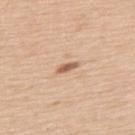<lesion>
  <image>
    <source>total-body photography crop</source>
    <field_of_view_mm>15</field_of_view_mm>
  </image>
  <site>upper back</site>
  <automated_metrics>
    <nevus_likeness_0_100>35</nevus_likeness_0_100>
  </automated_metrics>
  <lesion_size>
    <long_diameter_mm_approx>2.5</long_diameter_mm_approx>
  </lesion_size>
  <patient>
    <sex>male</sex>
    <age_approx>65</age_approx>
  </patient>
  <lighting>white-light</lighting>
</lesion>